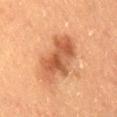Assessment:
The lesion was photographed on a routine skin check and not biopsied; there is no pathology result.